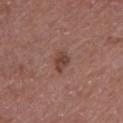No biopsy was performed on this lesion — it was imaged during a full skin examination and was not determined to be concerning. On the left upper arm. A close-up tile cropped from a whole-body skin photograph, about 15 mm across. A female patient, aged around 50.